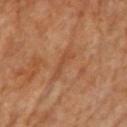<record>
  <biopsy_status>not biopsied; imaged during a skin examination</biopsy_status>
  <site>front of the torso</site>
  <image>
    <source>total-body photography crop</source>
    <field_of_view_mm>15</field_of_view_mm>
  </image>
  <patient>
    <sex>female</sex>
  </patient>
  <lesion_size>
    <long_diameter_mm_approx>4.0</long_diameter_mm_approx>
  </lesion_size>
  <automated_metrics>
    <color_variation_0_10>0.0</color_variation_0_10>
    <peripheral_color_asymmetry>0.0</peripheral_color_asymmetry>
  </automated_metrics>
  <lighting>cross-polarized</lighting>
</record>